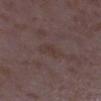Q: Was this lesion biopsied?
A: imaged on a skin check; not biopsied
Q: How was this image acquired?
A: ~15 mm tile from a whole-body skin photo
Q: Who is the patient?
A: female, in their mid-30s
Q: Lesion location?
A: the leg